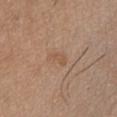- subject · male, aged 43–47
- lighting · white-light
- location · the front of the torso
- acquisition · total-body-photography crop, ~15 mm field of view